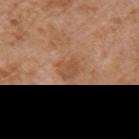{
  "biopsy_status": "not biopsied; imaged during a skin examination",
  "lighting": "white-light",
  "image": {
    "source": "total-body photography crop",
    "field_of_view_mm": 15
  },
  "lesion_size": {
    "long_diameter_mm_approx": 3.0
  },
  "site": "left upper arm",
  "automated_metrics": {
    "area_mm2_approx": 5.0,
    "eccentricity": 0.55
  },
  "patient": {
    "sex": "male",
    "age_approx": 75
  }
}